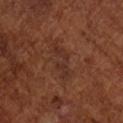TBP lesion metrics = a detector confidence of about 100 out of 100 that the crop contains a lesion; lesion size = about 4.5 mm; tile lighting = cross-polarized; imaging modality = ~15 mm crop, total-body skin-cancer survey; anatomic site = the arm; patient = male, aged approximately 65.